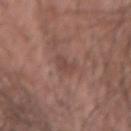Notes:
• acquisition — ~15 mm tile from a whole-body skin photo
• patient — male, aged 53–57
• lesion size — about 2.5 mm
• lighting — white-light
• site — the left forearm